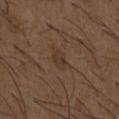Clinical impression: This lesion was catalogued during total-body skin photography and was not selected for biopsy. Context: This is a white-light tile. On the chest. A lesion tile, about 15 mm wide, cut from a 3D total-body photograph. The subject is a male approximately 50 years of age. The total-body-photography lesion software estimated a footprint of about 3.5 mm², a shape eccentricity near 0.8, and a shape-asymmetry score of about 0.25 (0 = symmetric). It also reported an average lesion color of about L≈34 a*≈15 b*≈24 (CIELAB), roughly 6 lightness units darker than nearby skin, and a lesion-to-skin contrast of about 5.5 (normalized; higher = more distinct). About 2.5 mm across.